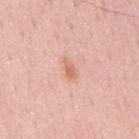Recorded during total-body skin imaging; not selected for excision or biopsy. Approximately 2.5 mm at its widest. The subject is a male approximately 50 years of age. An algorithmic analysis of the crop reported a border-irregularity rating of about 3/10, a within-lesion color-variation index near 2/10, and a peripheral color-asymmetry measure near 0.5. On the mid back. A close-up tile cropped from a whole-body skin photograph, about 15 mm across. The tile uses white-light illumination.